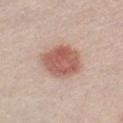Image and clinical context: Imaged with white-light lighting. The recorded lesion diameter is about 5 mm. A male patient, aged approximately 30. An algorithmic analysis of the crop reported a lesion area of about 15 mm², an outline eccentricity of about 0.55 (0 = round, 1 = elongated), and a symmetry-axis asymmetry near 0.15. The software also gave an average lesion color of about L≈58 a*≈23 b*≈27 (CIELAB), a lesion–skin lightness drop of about 13, and a normalized border contrast of about 9. The analysis additionally found a border-irregularity rating of about 1.5/10, internal color variation of about 4 on a 0–10 scale, and peripheral color asymmetry of about 1.5. The analysis additionally found a nevus-likeness score of about 100/100 and a detector confidence of about 100 out of 100 that the crop contains a lesion. A close-up tile cropped from a whole-body skin photograph, about 15 mm across.